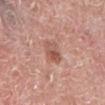Assessment:
Captured during whole-body skin photography for melanoma surveillance; the lesion was not biopsied.
Background:
Measured at roughly 2.5 mm in maximum diameter. This image is a 15 mm lesion crop taken from a total-body photograph. A male subject, approximately 65 years of age. This is a white-light tile. The lesion is on the left lower leg.